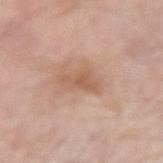{
  "site": "right upper arm",
  "lighting": "white-light",
  "lesion_size": {
    "long_diameter_mm_approx": 3.5
  },
  "image": {
    "source": "total-body photography crop",
    "field_of_view_mm": 15
  },
  "patient": {
    "sex": "male",
    "age_approx": 70
  }
}